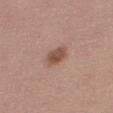The tile uses white-light illumination.
Automated image analysis of the tile measured border irregularity of about 2 on a 0–10 scale and peripheral color asymmetry of about 1. The software also gave an automated nevus-likeness rating near 85 out of 100 and a detector confidence of about 100 out of 100 that the crop contains a lesion.
About 3 mm across.
Cropped from a total-body skin-imaging series; the visible field is about 15 mm.
The lesion is on the right thigh.
A female subject, aged 38–42.